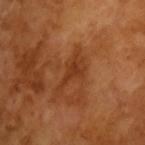  biopsy_status: not biopsied; imaged during a skin examination
  automated_metrics:
    border_irregularity_0_10: 7.5
    peripheral_color_asymmetry: 0.5
    nevus_likeness_0_100: 0
    lesion_detection_confidence_0_100: 100
  patient:
    sex: male
    age_approx: 65
  image:
    source: total-body photography crop
    field_of_view_mm: 15
  lighting: cross-polarized